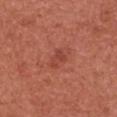  image:
    source: total-body photography crop
    field_of_view_mm: 15
  patient:
    sex: female
    age_approx: 50
  site: chest